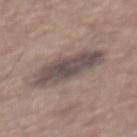workup = total-body-photography surveillance lesion; no biopsy
subject = male, about 60 years old
location = the upper back
image = ~15 mm crop, total-body skin-cancer survey
image-analysis metrics = a shape eccentricity near 0.9 and two-axis asymmetry of about 0.15; a lesion color around L≈48 a*≈12 b*≈17 in CIELAB and a normalized lesion–skin contrast near 9; a classifier nevus-likeness of about 5/100